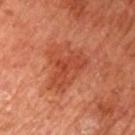Recorded during total-body skin imaging; not selected for excision or biopsy.
The lesion is on the arm.
The subject is a female aged around 65.
This image is a 15 mm lesion crop taken from a total-body photograph.
This is a cross-polarized tile.
The lesion's longest dimension is about 5.5 mm.